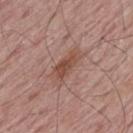notes = no biopsy performed (imaged during a skin exam)
subject = male, aged 63 to 67
acquisition = 15 mm crop, total-body photography
tile lighting = white-light illumination
anatomic site = the upper back